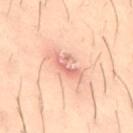Q: Was a biopsy performed?
A: catalogued during a skin exam; not biopsied
Q: How was the tile lit?
A: cross-polarized illumination
Q: What is the imaging modality?
A: total-body-photography crop, ~15 mm field of view
Q: What are the patient's age and sex?
A: male, about 30 years old
Q: Where on the body is the lesion?
A: the right thigh